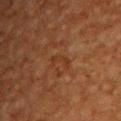workup: total-body-photography surveillance lesion; no biopsy | lighting: cross-polarized illumination | diameter: ≈3 mm | site: the upper back | patient: male, aged 48 to 52 | automated lesion analysis: an eccentricity of roughly 0.9 and a shape-asymmetry score of about 0.55 (0 = symmetric); a mean CIELAB color near L≈27 a*≈19 b*≈27, a lesion–skin lightness drop of about 4, and a normalized border contrast of about 5; a border-irregularity rating of about 6/10 and a color-variation rating of about 0/10; a lesion-detection confidence of about 100/100 | acquisition: ~15 mm tile from a whole-body skin photo.